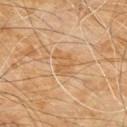  image:
    source: total-body photography crop
    field_of_view_mm: 15
  site: chest
  lighting: cross-polarized
  patient:
    sex: male
    age_approx: 60
  lesion_size:
    long_diameter_mm_approx: 3.0